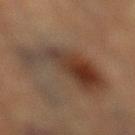biopsy_status: not biopsied; imaged during a skin examination
image:
  source: total-body photography crop
  field_of_view_mm: 15
lesion_size:
  long_diameter_mm_approx: 8.5
patient:
  sex: male
  age_approx: 85+
site: left lower leg
automated_metrics:
  nevus_likeness_0_100: 100
  lesion_detection_confidence_0_100: 100